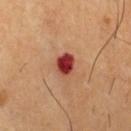follow-up = catalogued during a skin exam; not biopsied | patient = male, approximately 60 years of age | image source = ~15 mm tile from a whole-body skin photo | size = ~3 mm (longest diameter) | location = the chest | lighting = cross-polarized illumination | image-analysis metrics = an area of roughly 6 mm² and an eccentricity of roughly 0.55; a border-irregularity index near 2/10 and a within-lesion color-variation index near 5/10.